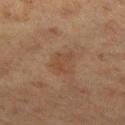Q: Is there a histopathology result?
A: imaged on a skin check; not biopsied
Q: Illumination type?
A: cross-polarized
Q: Lesion location?
A: the left thigh
Q: How was this image acquired?
A: 15 mm crop, total-body photography
Q: What did automated image analysis measure?
A: an average lesion color of about L≈38 a*≈17 b*≈26 (CIELAB), roughly 5 lightness units darker than nearby skin, and a normalized border contrast of about 5; border irregularity of about 3.5 on a 0–10 scale, a within-lesion color-variation index near 2.5/10, and a peripheral color-asymmetry measure near 1
Q: What are the patient's age and sex?
A: female, about 40 years old
Q: What is the lesion's diameter?
A: about 3 mm